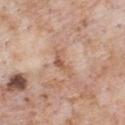Recorded during total-body skin imaging; not selected for excision or biopsy. A lesion tile, about 15 mm wide, cut from a 3D total-body photograph. A male subject aged 63–67. The lesion is located on the front of the torso.